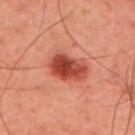Imaged during a routine full-body skin examination; the lesion was not biopsied and no histopathology is available.
A male subject aged approximately 45.
From the right upper arm.
This image is a 15 mm lesion crop taken from a total-body photograph.